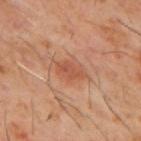Approximately 3.5 mm at its widest.
Cropped from a total-body skin-imaging series; the visible field is about 15 mm.
A male patient, approximately 60 years of age.
The lesion is on the mid back.
Imaged with cross-polarized lighting.
Automated image analysis of the tile measured a footprint of about 5.5 mm², an eccentricity of roughly 0.8, and two-axis asymmetry of about 0.3. The analysis additionally found a lesion–skin lightness drop of about 7. It also reported a border-irregularity rating of about 3/10 and peripheral color asymmetry of about 1. The software also gave a nevus-likeness score of about 80/100 and a lesion-detection confidence of about 100/100.